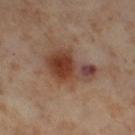<record>
  <biopsy_status>not biopsied; imaged during a skin examination</biopsy_status>
  <patient>
    <sex>female</sex>
    <age_approx>55</age_approx>
  </patient>
  <image>
    <source>total-body photography crop</source>
    <field_of_view_mm>15</field_of_view_mm>
  </image>
  <lighting>cross-polarized</lighting>
  <lesion_size>
    <long_diameter_mm_approx>5.5</long_diameter_mm_approx>
  </lesion_size>
  <site>leg</site>
</record>